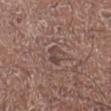<case>
  <biopsy_status>not biopsied; imaged during a skin examination</biopsy_status>
  <image>
    <source>total-body photography crop</source>
    <field_of_view_mm>15</field_of_view_mm>
  </image>
  <lighting>white-light</lighting>
  <patient>
    <sex>female</sex>
    <age_approx>50</age_approx>
  </patient>
  <site>right lower leg</site>
  <automated_metrics>
    <cielab_L>43</cielab_L>
    <cielab_a>17</cielab_a>
    <cielab_b>21</cielab_b>
    <vs_skin_contrast_norm>6.0</vs_skin_contrast_norm>
    <border_irregularity_0_10>4.0</border_irregularity_0_10>
    <color_variation_0_10>0.5</color_variation_0_10>
    <peripheral_color_asymmetry>0.0</peripheral_color_asymmetry>
    <lesion_detection_confidence_0_100>65</lesion_detection_confidence_0_100>
  </automated_metrics>
  <lesion_size>
    <long_diameter_mm_approx>2.5</long_diameter_mm_approx>
  </lesion_size>
</case>